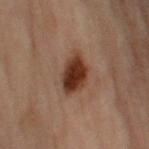Approximately 4.5 mm at its widest.
Imaged with cross-polarized lighting.
A male subject aged 68–72.
From the left upper arm.
Automated image analysis of the tile measured a lesion color around L≈24 a*≈17 b*≈22 in CIELAB, about 13 CIELAB-L* units darker than the surrounding skin, and a lesion-to-skin contrast of about 13.5 (normalized; higher = more distinct).
A 15 mm close-up tile from a total-body photography series done for melanoma screening.